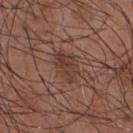location=the chest
image=total-body-photography crop, ~15 mm field of view
diameter=≈4 mm
tile lighting=white-light illumination
TBP lesion metrics=a lesion area of about 8 mm², an eccentricity of roughly 0.8, and two-axis asymmetry of about 0.25; a lesion–skin lightness drop of about 7 and a normalized lesion–skin contrast near 6.5; a classifier nevus-likeness of about 10/100 and a detector confidence of about 95 out of 100 that the crop contains a lesion
subject=male, approximately 55 years of age